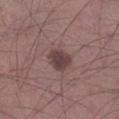Imaged during a routine full-body skin examination; the lesion was not biopsied and no histopathology is available.
The tile uses white-light illumination.
From the leg.
The lesion's longest dimension is about 3 mm.
A close-up tile cropped from a whole-body skin photograph, about 15 mm across.
Automated tile analysis of the lesion measured an area of roughly 6.5 mm², an outline eccentricity of about 0.55 (0 = round, 1 = elongated), and two-axis asymmetry of about 0.25. The software also gave roughly 11 lightness units darker than nearby skin and a normalized lesion–skin contrast near 9. The software also gave a within-lesion color-variation index near 2/10 and a peripheral color-asymmetry measure near 0.5. It also reported a classifier nevus-likeness of about 35/100 and a lesion-detection confidence of about 100/100.
The subject is a male in their mid- to late 30s.